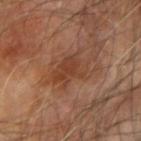Imaged during a routine full-body skin examination; the lesion was not biopsied and no histopathology is available.
On the left forearm.
The tile uses cross-polarized illumination.
A male subject, aged around 70.
Approximately 4.5 mm at its widest.
A region of skin cropped from a whole-body photographic capture, roughly 15 mm wide.